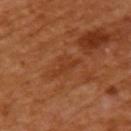No biopsy was performed on this lesion — it was imaged during a full skin examination and was not determined to be concerning. The lesion is located on the arm. The recorded lesion diameter is about 3.5 mm. Imaged with cross-polarized lighting. A female subject in their mid-50s. Cropped from a total-body skin-imaging series; the visible field is about 15 mm.